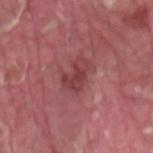* notes: imaged on a skin check; not biopsied